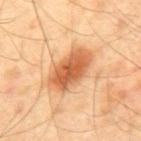Q: Was this lesion biopsied?
A: no biopsy performed (imaged during a skin exam)
Q: Who is the patient?
A: male, aged approximately 40
Q: How was the tile lit?
A: cross-polarized
Q: Automated lesion metrics?
A: a border-irregularity index near 2/10, a within-lesion color-variation index near 5.5/10, and peripheral color asymmetry of about 1.5
Q: How large is the lesion?
A: ≈6 mm
Q: How was this image acquired?
A: total-body-photography crop, ~15 mm field of view
Q: What is the anatomic site?
A: the mid back